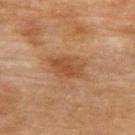A female patient, about 80 years old. The lesion is located on the back. Imaged with cross-polarized lighting. Approximately 4.5 mm at its widest. A 15 mm crop from a total-body photograph taken for skin-cancer surveillance.